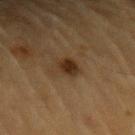Recorded during total-body skin imaging; not selected for excision or biopsy.
A roughly 15 mm field-of-view crop from a total-body skin photograph.
A male patient, about 85 years old or older.
This is a cross-polarized tile.
Longest diameter approximately 3 mm.
On the left upper arm.